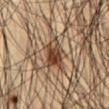<case>
  <biopsy_status>not biopsied; imaged during a skin examination</biopsy_status>
  <patient>
    <sex>male</sex>
    <age_approx>50</age_approx>
  </patient>
  <site>chest</site>
  <lighting>cross-polarized</lighting>
  <automated_metrics>
    <area_mm2_approx>11.0</area_mm2_approx>
    <eccentricity>0.6</eccentricity>
    <shape_asymmetry>0.35</shape_asymmetry>
    <cielab_L>43</cielab_L>
    <cielab_a>16</cielab_a>
    <cielab_b>29</cielab_b>
    <vs_skin_contrast_norm>9.5</vs_skin_contrast_norm>
  </automated_metrics>
  <image>
    <source>total-body photography crop</source>
    <field_of_view_mm>15</field_of_view_mm>
  </image>
  <lesion_size>
    <long_diameter_mm_approx>4.0</long_diameter_mm_approx>
  </lesion_size>
</case>